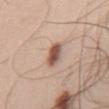notes: total-body-photography surveillance lesion; no biopsy
site: the chest
automated metrics: a lesion area of about 6 mm² and a shape eccentricity near 0.8; an average lesion color of about L≈55 a*≈20 b*≈27 (CIELAB), a lesion–skin lightness drop of about 15, and a normalized lesion–skin contrast near 10; a border-irregularity index near 2/10, a color-variation rating of about 6.5/10, and peripheral color asymmetry of about 2
subject: male, about 55 years old
lesion diameter: about 3.5 mm
illumination: white-light
image source: ~15 mm crop, total-body skin-cancer survey